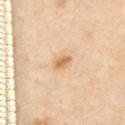Assessment: No biopsy was performed on this lesion — it was imaged during a full skin examination and was not determined to be concerning. Context: A female patient, aged 53 to 57. The lesion is on the chest. A roughly 15 mm field-of-view crop from a total-body skin photograph.